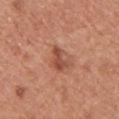The lesion is located on the chest.
A female subject aged 33 to 37.
Imaged with white-light lighting.
About 3.5 mm across.
Automated image analysis of the tile measured an outline eccentricity of about 0.8 (0 = round, 1 = elongated) and a symmetry-axis asymmetry near 0.4. The software also gave an automated nevus-likeness rating near 0 out of 100 and lesion-presence confidence of about 100/100.
Cropped from a total-body skin-imaging series; the visible field is about 15 mm.